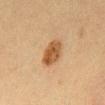Q: Was a biopsy performed?
A: total-body-photography surveillance lesion; no biopsy
Q: Patient demographics?
A: female, aged 38 to 42
Q: What kind of image is this?
A: ~15 mm crop, total-body skin-cancer survey
Q: How was the tile lit?
A: cross-polarized illumination
Q: Lesion location?
A: the front of the torso
Q: Automated lesion metrics?
A: a footprint of about 7.5 mm², an eccentricity of roughly 0.75, and a shape-asymmetry score of about 0.15 (0 = symmetric); a border-irregularity index near 1.5/10, internal color variation of about 4 on a 0–10 scale, and radial color variation of about 1.5; an automated nevus-likeness rating near 95 out of 100
Q: What is the lesion's diameter?
A: about 3.5 mm